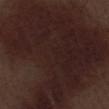follow-up: catalogued during a skin exam; not biopsied | image-analysis metrics: an area of roughly 175 mm², a shape eccentricity near 0.6, and a symmetry-axis asymmetry near 0.55; a mean CIELAB color near L≈20 a*≈16 b*≈16, roughly 9 lightness units darker than nearby skin, and a lesion-to-skin contrast of about 10.5 (normalized; higher = more distinct); a border-irregularity index near 7.5/10, a within-lesion color-variation index near 3.5/10, and radial color variation of about 1; a nevus-likeness score of about 5/100 and a lesion-detection confidence of about 75/100 | tile lighting: white-light | lesion diameter: ≈19.5 mm | body site: the right lower leg | imaging modality: total-body-photography crop, ~15 mm field of view | subject: male, aged around 70.